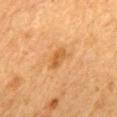<tbp_lesion>
  <biopsy_status>not biopsied; imaged during a skin examination</biopsy_status>
  <image>
    <source>total-body photography crop</source>
    <field_of_view_mm>15</field_of_view_mm>
  </image>
  <patient>
    <sex>female</sex>
    <age_approx>55</age_approx>
  </patient>
  <lighting>cross-polarized</lighting>
  <site>back</site>
</tbp_lesion>